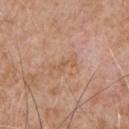notes=catalogued during a skin exam; not biopsied
acquisition=total-body-photography crop, ~15 mm field of view
location=the chest
patient=male, aged 63 to 67